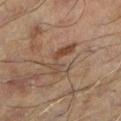Notes:
• follow-up · imaged on a skin check; not biopsied
• subject · male, roughly 60 years of age
• anatomic site · the left lower leg
• illumination · cross-polarized illumination
• TBP lesion metrics · a footprint of about 8 mm² and a symmetry-axis asymmetry near 0.6; an average lesion color of about L≈34 a*≈13 b*≈22 (CIELAB) and a lesion-to-skin contrast of about 6 (normalized; higher = more distinct)
• imaging modality · ~15 mm crop, total-body skin-cancer survey